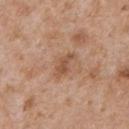Q: Is there a histopathology result?
A: catalogued during a skin exam; not biopsied
Q: Patient demographics?
A: male, in their mid-60s
Q: Where on the body is the lesion?
A: the chest
Q: How was this image acquired?
A: ~15 mm tile from a whole-body skin photo